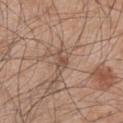– workup · total-body-photography surveillance lesion; no biopsy
– location · the right upper arm
– subject · male, aged around 45
– image source · total-body-photography crop, ~15 mm field of view